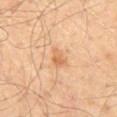Case summary:
- biopsy status — catalogued during a skin exam; not biopsied
- patient — male, in their mid-50s
- site — the lower back
- acquisition — total-body-photography crop, ~15 mm field of view
- automated lesion analysis — a lesion color around L≈61 a*≈22 b*≈37 in CIELAB, a lesion–skin lightness drop of about 9, and a lesion-to-skin contrast of about 6 (normalized; higher = more distinct)